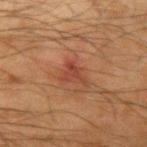The lesion was tiled from a total-body skin photograph and was not biopsied.